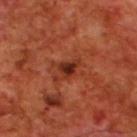Impression: The lesion was tiled from a total-body skin photograph and was not biopsied. Context: A male patient about 70 years old. The lesion is located on the upper back. This image is a 15 mm lesion crop taken from a total-body photograph.